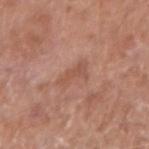| feature | finding |
|---|---|
| workup | catalogued during a skin exam; not biopsied |
| lighting | white-light |
| body site | the right upper arm |
| size | ~3.5 mm (longest diameter) |
| imaging modality | ~15 mm tile from a whole-body skin photo |
| patient | male, aged approximately 65 |